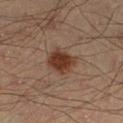Q: What is the lesion's diameter?
A: ~3.5 mm (longest diameter)
Q: What is the imaging modality?
A: ~15 mm crop, total-body skin-cancer survey
Q: What are the patient's age and sex?
A: male, aged 38–42
Q: What is the anatomic site?
A: the left lower leg
Q: What did automated image analysis measure?
A: a lesion area of about 8.5 mm², an eccentricity of roughly 0.45, and two-axis asymmetry of about 0.2; an average lesion color of about L≈32 a*≈18 b*≈25 (CIELAB), roughly 11 lightness units darker than nearby skin, and a normalized border contrast of about 10.5; border irregularity of about 2 on a 0–10 scale, a color-variation rating of about 2.5/10, and peripheral color asymmetry of about 1; lesion-presence confidence of about 100/100
Q: What lighting was used for the tile?
A: cross-polarized